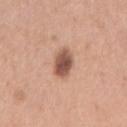<lesion>
<biopsy_status>not biopsied; imaged during a skin examination</biopsy_status>
<automated_metrics>
  <area_mm2_approx>7.5</area_mm2_approx>
  <eccentricity>0.8</eccentricity>
  <cielab_L>54</cielab_L>
  <cielab_a>22</cielab_a>
  <cielab_b>28</cielab_b>
  <nevus_likeness_0_100>95</nevus_likeness_0_100>
  <lesion_detection_confidence_0_100>100</lesion_detection_confidence_0_100>
</automated_metrics>
<site>right upper arm</site>
<patient>
  <sex>female</sex>
  <age_approx>55</age_approx>
</patient>
<image>
  <source>total-body photography crop</source>
  <field_of_view_mm>15</field_of_view_mm>
</image>
</lesion>